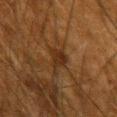This lesion was catalogued during total-body skin photography and was not selected for biopsy. Imaged with cross-polarized lighting. A male patient, aged 63 to 67. Measured at roughly 3 mm in maximum diameter. A 15 mm crop from a total-body photograph taken for skin-cancer surveillance. The lesion is on the right upper arm.